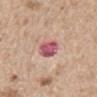| field | value |
|---|---|
| notes | catalogued during a skin exam; not biopsied |
| patient | male, aged 68–72 |
| lesion size | ≈3.5 mm |
| TBP lesion metrics | a classifier nevus-likeness of about 0/100 |
| body site | the back |
| imaging modality | ~15 mm tile from a whole-body skin photo |